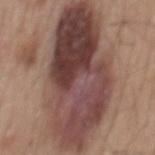Case summary:
– notes — no biopsy performed (imaged during a skin exam)
– automated lesion analysis — a footprint of about 80 mm², an eccentricity of roughly 0.9, and two-axis asymmetry of about 0.15; an average lesion color of about L≈44 a*≈19 b*≈21 (CIELAB) and about 15 CIELAB-L* units darker than the surrounding skin; border irregularity of about 3 on a 0–10 scale and internal color variation of about 9.5 on a 0–10 scale; lesion-presence confidence of about 100/100
– location — the mid back
– patient — male, aged approximately 55
– illumination — white-light
– lesion diameter — ≈16 mm
– image source — total-body-photography crop, ~15 mm field of view